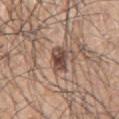biopsy_status: not biopsied; imaged during a skin examination
lighting: white-light
site: left arm
image:
  source: total-body photography crop
  field_of_view_mm: 15
automated_metrics:
  area_mm2_approx: 6.0
  eccentricity: 0.55
  shape_asymmetry: 0.2
  border_irregularity_0_10: 2.0
  color_variation_0_10: 4.5
  peripheral_color_asymmetry: 1.5
patient:
  sex: male
  age_approx: 70